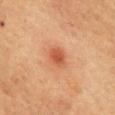Clinical summary: The lesion is located on the back. A 15 mm close-up extracted from a 3D total-body photography capture. An algorithmic analysis of the crop reported an area of roughly 5 mm², an outline eccentricity of about 0.7 (0 = round, 1 = elongated), and a shape-asymmetry score of about 0.15 (0 = symmetric). And it measured a lesion color around L≈49 a*≈28 b*≈34 in CIELAB, a lesion–skin lightness drop of about 10, and a normalized lesion–skin contrast near 7. Imaged with cross-polarized lighting. A female patient, in their 60s.